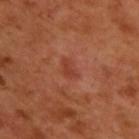Part of a total-body skin-imaging series; this lesion was reviewed on a skin check and was not flagged for biopsy.
The total-body-photography lesion software estimated a lesion color around L≈41 a*≈30 b*≈32 in CIELAB, roughly 7 lightness units darker than nearby skin, and a normalized border contrast of about 5.5. The analysis additionally found a nevus-likeness score of about 25/100 and a detector confidence of about 100 out of 100 that the crop contains a lesion.
A 15 mm crop from a total-body photograph taken for skin-cancer surveillance.
The patient is a male in their 50s.
From the back.
Approximately 2.5 mm at its widest.